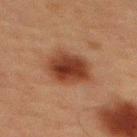Part of a total-body skin-imaging series; this lesion was reviewed on a skin check and was not flagged for biopsy. The lesion is on the upper back. A lesion tile, about 15 mm wide, cut from a 3D total-body photograph. A male patient, roughly 60 years of age.